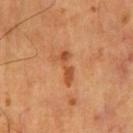image source — ~15 mm crop, total-body skin-cancer survey | diameter — ≈4 mm | patient — male, approximately 55 years of age | illumination — cross-polarized | body site — the chest.